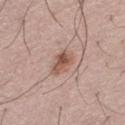Clinical impression: Captured during whole-body skin photography for melanoma surveillance; the lesion was not biopsied. Context: Imaged with white-light lighting. This image is a 15 mm lesion crop taken from a total-body photograph. The subject is a male in their mid- to late 50s. Automated image analysis of the tile measured a footprint of about 5.5 mm² and two-axis asymmetry of about 0.3. The software also gave border irregularity of about 3 on a 0–10 scale, a within-lesion color-variation index near 6.5/10, and peripheral color asymmetry of about 3.